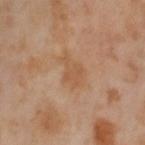Impression:
Captured during whole-body skin photography for melanoma surveillance; the lesion was not biopsied.
Clinical summary:
Located on the left thigh. The tile uses cross-polarized illumination. Longest diameter approximately 4.5 mm. The subject is a female aged approximately 55. A close-up tile cropped from a whole-body skin photograph, about 15 mm across.